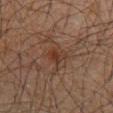Imaged during a routine full-body skin examination; the lesion was not biopsied and no histopathology is available. The lesion is on the abdomen. Captured under cross-polarized illumination. The patient is a male in their 60s. Longest diameter approximately 2.5 mm. A lesion tile, about 15 mm wide, cut from a 3D total-body photograph.